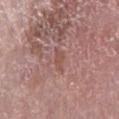{"automated_metrics": {"eccentricity": 0.85, "vs_skin_darker_L": 6.0, "vs_skin_contrast_norm": 5.0}, "site": "left lower leg", "image": {"source": "total-body photography crop", "field_of_view_mm": 15}, "lighting": "white-light", "patient": {"sex": "female", "age_approx": 60}}